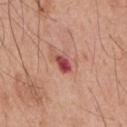biopsy status: imaged on a skin check; not biopsied | subject: male, aged 53 to 57 | tile lighting: white-light illumination | anatomic site: the front of the torso | diameter: ~2.5 mm (longest diameter) | imaging modality: ~15 mm crop, total-body skin-cancer survey.